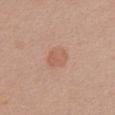Findings:
• lesion diameter: ~3 mm (longest diameter)
• body site: the chest
• patient: female, aged 33 to 37
• tile lighting: white-light
• image source: ~15 mm crop, total-body skin-cancer survey